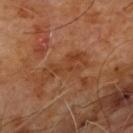<case>
  <biopsy_status>not biopsied; imaged during a skin examination</biopsy_status>
  <lesion_size>
    <long_diameter_mm_approx>5.5</long_diameter_mm_approx>
  </lesion_size>
  <image>
    <source>total-body photography crop</source>
    <field_of_view_mm>15</field_of_view_mm>
  </image>
  <site>chest</site>
  <lighting>cross-polarized</lighting>
  <automated_metrics>
    <area_mm2_approx>8.0</area_mm2_approx>
    <shape_asymmetry>0.5</shape_asymmetry>
    <cielab_L>38</cielab_L>
    <cielab_a>23</cielab_a>
    <cielab_b>33</cielab_b>
    <vs_skin_darker_L>6.0</vs_skin_darker_L>
    <vs_skin_contrast_norm>6.0</vs_skin_contrast_norm>
    <border_irregularity_0_10>7.5</border_irregularity_0_10>
    <peripheral_color_asymmetry>1.0</peripheral_color_asymmetry>
    <lesion_detection_confidence_0_100>100</lesion_detection_confidence_0_100>
  </automated_metrics>
  <patient>
    <sex>male</sex>
    <age_approx>60</age_approx>
  </patient>
</case>